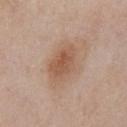Findings:
* workup: no biopsy performed (imaged during a skin exam)
* patient: male, aged around 65
* imaging modality: ~15 mm crop, total-body skin-cancer survey
* anatomic site: the front of the torso